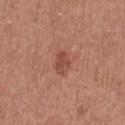{
  "lesion_size": {
    "long_diameter_mm_approx": 3.0
  },
  "automated_metrics": {
    "vs_skin_darker_L": 9.0,
    "vs_skin_contrast_norm": 6.5,
    "peripheral_color_asymmetry": 0.5,
    "nevus_likeness_0_100": 10,
    "lesion_detection_confidence_0_100": 100
  },
  "image": {
    "source": "total-body photography crop",
    "field_of_view_mm": 15
  },
  "site": "leg",
  "patient": {
    "sex": "female",
    "age_approx": 50
  }
}